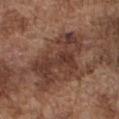Part of a total-body skin-imaging series; this lesion was reviewed on a skin check and was not flagged for biopsy. About 7 mm across. From the front of the torso. A lesion tile, about 15 mm wide, cut from a 3D total-body photograph. The patient is a male about 75 years old. An algorithmic analysis of the crop reported a footprint of about 17 mm², an eccentricity of roughly 0.85, and two-axis asymmetry of about 0.55. The software also gave a mean CIELAB color near L≈36 a*≈20 b*≈24 and a normalized lesion–skin contrast near 9. It also reported a color-variation rating of about 4/10. The software also gave an automated nevus-likeness rating near 0 out of 100 and lesion-presence confidence of about 90/100. The tile uses white-light illumination.